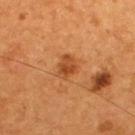<tbp_lesion>
  <biopsy_status>not biopsied; imaged during a skin examination</biopsy_status>
  <lighting>cross-polarized</lighting>
  <lesion_size>
    <long_diameter_mm_approx>3.0</long_diameter_mm_approx>
  </lesion_size>
  <automated_metrics>
    <area_mm2_approx>5.0</area_mm2_approx>
    <eccentricity>0.6</eccentricity>
    <cielab_L>44</cielab_L>
    <cielab_a>27</cielab_a>
    <cielab_b>39</cielab_b>
    <vs_skin_darker_L>10.0</vs_skin_darker_L>
    <vs_skin_contrast_norm>7.5</vs_skin_contrast_norm>
    <border_irregularity_0_10>2.5</border_irregularity_0_10>
    <peripheral_color_asymmetry>1.5</peripheral_color_asymmetry>
    <nevus_likeness_0_100>85</nevus_likeness_0_100>
    <lesion_detection_confidence_0_100>100</lesion_detection_confidence_0_100>
  </automated_metrics>
  <patient>
    <sex>male</sex>
    <age_approx>55</age_approx>
  </patient>
  <site>upper back</site>
  <image>
    <source>total-body photography crop</source>
    <field_of_view_mm>15</field_of_view_mm>
  </image>
</tbp_lesion>